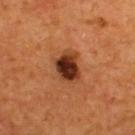<lesion>
<biopsy_status>not biopsied; imaged during a skin examination</biopsy_status>
<patient>
  <sex>male</sex>
  <age_approx>55</age_approx>
</patient>
<image>
  <source>total-body photography crop</source>
  <field_of_view_mm>15</field_of_view_mm>
</image>
<site>back</site>
</lesion>